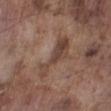Assessment: The lesion was tiled from a total-body skin photograph and was not biopsied. Image and clinical context: The lesion is located on the left lower leg. The patient is a male in their mid-70s. This image is a 15 mm lesion crop taken from a total-body photograph. Imaged with white-light lighting.